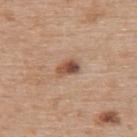Recorded during total-body skin imaging; not selected for excision or biopsy. A 15 mm close-up tile from a total-body photography series done for melanoma screening. The tile uses white-light illumination. A female subject, aged 63 to 67. From the upper back. Approximately 3 mm at its widest.